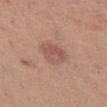Clinical impression: Recorded during total-body skin imaging; not selected for excision or biopsy. Clinical summary: The total-body-photography lesion software estimated a lesion area of about 7 mm² and a symmetry-axis asymmetry near 0.2. It also reported a lesion–skin lightness drop of about 8 and a normalized lesion–skin contrast near 6. The software also gave a classifier nevus-likeness of about 15/100. A 15 mm close-up extracted from a 3D total-body photography capture. The tile uses white-light illumination. From the left thigh. The subject is a female in their 40s. Measured at roughly 3 mm in maximum diameter.